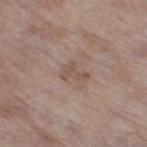Impression:
Recorded during total-body skin imaging; not selected for excision or biopsy.